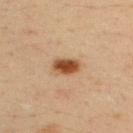Case summary:
– follow-up · total-body-photography surveillance lesion; no biopsy
– illumination · cross-polarized illumination
– body site · the back
– imaging modality · total-body-photography crop, ~15 mm field of view
– subject · male, aged approximately 35